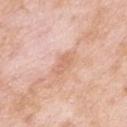biopsy_status: not biopsied; imaged during a skin examination
site: left upper arm
patient:
  sex: male
  age_approx: 55
lesion_size:
  long_diameter_mm_approx: 3.0
lighting: white-light
image:
  source: total-body photography crop
  field_of_view_mm: 15